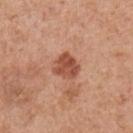Notes:
– biopsy status · catalogued during a skin exam; not biopsied
– anatomic site · the chest
– size · ~3 mm (longest diameter)
– patient · male, aged 53–57
– acquisition · ~15 mm crop, total-body skin-cancer survey
– tile lighting · white-light
– automated metrics · a lesion area of about 7.5 mm², a shape eccentricity near 0.15, and a shape-asymmetry score of about 0.25 (0 = symmetric); a border-irregularity index near 2.5/10 and radial color variation of about 1.5